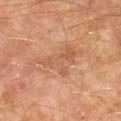{
  "biopsy_status": "not biopsied; imaged during a skin examination",
  "patient": {
    "sex": "male",
    "age_approx": 60
  },
  "image": {
    "source": "total-body photography crop",
    "field_of_view_mm": 15
  },
  "lighting": "cross-polarized",
  "site": "leg"
}